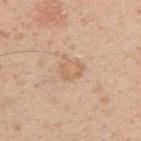Assessment:
No biopsy was performed on this lesion — it was imaged during a full skin examination and was not determined to be concerning.
Background:
Captured under white-light illumination. A 15 mm close-up tile from a total-body photography series done for melanoma screening. A male patient approximately 30 years of age. The lesion is on the right upper arm. Approximately 3 mm at its widest.